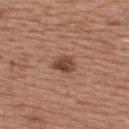| feature | finding |
|---|---|
| follow-up | imaged on a skin check; not biopsied |
| body site | the upper back |
| imaging modality | ~15 mm tile from a whole-body skin photo |
| lesion diameter | ≈2.5 mm |
| subject | female, aged 38 to 42 |
| lighting | white-light |